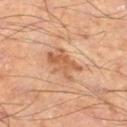notes = imaged on a skin check; not biopsied | body site = the leg | subject = male, in their mid-50s | image = 15 mm crop, total-body photography.